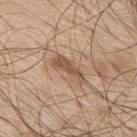{"biopsy_status": "not biopsied; imaged during a skin examination", "lighting": "white-light", "lesion_size": {"long_diameter_mm_approx": 4.5}, "site": "upper back", "image": {"source": "total-body photography crop", "field_of_view_mm": 15}, "patient": {"sex": "male", "age_approx": 70}, "automated_metrics": {"area_mm2_approx": 6.5, "eccentricity": 0.95, "shape_asymmetry": 0.35, "nevus_likeness_0_100": 5, "lesion_detection_confidence_0_100": 95}}